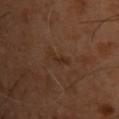follow-up: imaged on a skin check; not biopsied
anatomic site: the chest
imaging modality: ~15 mm tile from a whole-body skin photo
patient: male, aged 53 to 57